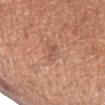No biopsy was performed on this lesion — it was imaged during a full skin examination and was not determined to be concerning. The subject is a female approximately 65 years of age. This image is a 15 mm lesion crop taken from a total-body photograph. From the right forearm.